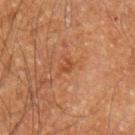Q: Who is the patient?
A: male, aged around 60
Q: What is the lesion's diameter?
A: about 1.5 mm
Q: What is the imaging modality?
A: ~15 mm tile from a whole-body skin photo
Q: What is the anatomic site?
A: the arm
Q: What lighting was used for the tile?
A: cross-polarized illumination
Q: Automated lesion metrics?
A: a footprint of about 1.5 mm², a shape eccentricity near 0.6, and a symmetry-axis asymmetry near 0.45